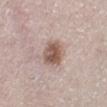Q: Was this lesion biopsied?
A: no biopsy performed (imaged during a skin exam)
Q: Automated lesion metrics?
A: a nevus-likeness score of about 90/100 and lesion-presence confidence of about 100/100
Q: What kind of image is this?
A: total-body-photography crop, ~15 mm field of view
Q: Patient demographics?
A: female, aged 53–57
Q: What is the anatomic site?
A: the left lower leg
Q: How was the tile lit?
A: white-light